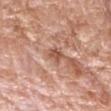image — total-body-photography crop, ~15 mm field of view | lesion diameter — about 3 mm | patient — male, about 75 years old | automated metrics — a mean CIELAB color near L≈55 a*≈23 b*≈30, roughly 11 lightness units darker than nearby skin, and a lesion-to-skin contrast of about 7.5 (normalized; higher = more distinct); a lesion-detection confidence of about 85/100 | illumination — white-light illumination | site — the head or neck.